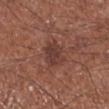biopsy status = catalogued during a skin exam; not biopsied
acquisition = ~15 mm tile from a whole-body skin photo
lesion size = about 3.5 mm
lighting = white-light
body site = the right lower leg
subject = male, aged approximately 70